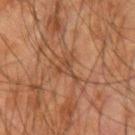Clinical impression:
This lesion was catalogued during total-body skin photography and was not selected for biopsy.
Acquisition and patient details:
The lesion is located on the right forearm. Captured under cross-polarized illumination. A male subject aged 63 to 67. The lesion's longest dimension is about 3.5 mm. A region of skin cropped from a whole-body photographic capture, roughly 15 mm wide.